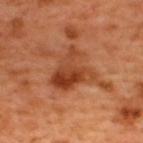No biopsy was performed on this lesion — it was imaged during a full skin examination and was not determined to be concerning. The patient is a male aged 48 to 52. The tile uses cross-polarized illumination. This image is a 15 mm lesion crop taken from a total-body photograph. The lesion is located on the back. An algorithmic analysis of the crop reported a footprint of about 14 mm² and a shape eccentricity near 0.55. The analysis additionally found a border-irregularity index near 5.5/10 and a peripheral color-asymmetry measure near 3.5. And it measured a classifier nevus-likeness of about 10/100 and lesion-presence confidence of about 100/100. Longest diameter approximately 5 mm.